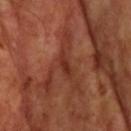- notes — total-body-photography surveillance lesion; no biopsy
- lesion size — about 3 mm
- image — 15 mm crop, total-body photography
- image-analysis metrics — a lesion color around L≈32 a*≈26 b*≈30 in CIELAB, a lesion–skin lightness drop of about 8, and a lesion-to-skin contrast of about 7.5 (normalized; higher = more distinct); a nevus-likeness score of about 0/100 and a detector confidence of about 55 out of 100 that the crop contains a lesion
- anatomic site — the head or neck
- lighting — cross-polarized illumination
- subject — male, aged 68 to 72